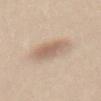{
  "biopsy_status": "not biopsied; imaged during a skin examination",
  "automated_metrics": {
    "cielab_L": 62,
    "cielab_a": 16,
    "cielab_b": 27,
    "vs_skin_darker_L": 10.0,
    "vs_skin_contrast_norm": 6.5
  },
  "lesion_size": {
    "long_diameter_mm_approx": 4.0
  },
  "patient": {
    "sex": "female",
    "age_approx": 45
  },
  "image": {
    "source": "total-body photography crop",
    "field_of_view_mm": 15
  },
  "site": "abdomen"
}